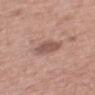Q: Is there a histopathology result?
A: no biopsy performed (imaged during a skin exam)
Q: Automated lesion metrics?
A: an average lesion color of about L≈53 a*≈20 b*≈23 (CIELAB), a lesion–skin lightness drop of about 11, and a lesion-to-skin contrast of about 7.5 (normalized; higher = more distinct); a border-irregularity rating of about 2.5/10, a within-lesion color-variation index near 2/10, and peripheral color asymmetry of about 0.5; a classifier nevus-likeness of about 40/100 and a detector confidence of about 100 out of 100 that the crop contains a lesion
Q: Illumination type?
A: white-light
Q: What is the lesion's diameter?
A: ≈3.5 mm
Q: What kind of image is this?
A: total-body-photography crop, ~15 mm field of view
Q: What are the patient's age and sex?
A: male, roughly 75 years of age
Q: What is the anatomic site?
A: the mid back